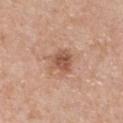{
  "biopsy_status": "not biopsied; imaged during a skin examination",
  "patient": {
    "sex": "male",
    "age_approx": 50
  },
  "site": "chest",
  "image": {
    "source": "total-body photography crop",
    "field_of_view_mm": 15
  }
}